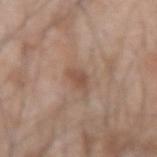workup = total-body-photography surveillance lesion; no biopsy | acquisition = ~15 mm tile from a whole-body skin photo | tile lighting = white-light illumination | diameter = ~2.5 mm (longest diameter) | patient = male, in their mid-40s | anatomic site = the left forearm.